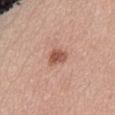The lesion is located on the abdomen.
A close-up tile cropped from a whole-body skin photograph, about 15 mm across.
Longest diameter approximately 3 mm.
Imaged with white-light lighting.
A female patient, in their 40s.